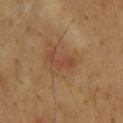| key | value |
|---|---|
| biopsy status | imaged on a skin check; not biopsied |
| tile lighting | cross-polarized illumination |
| lesion diameter | ~4 mm (longest diameter) |
| anatomic site | the upper back |
| imaging modality | total-body-photography crop, ~15 mm field of view |
| subject | male, roughly 65 years of age |